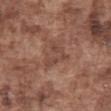biopsy status: total-body-photography surveillance lesion; no biopsy | site: the abdomen | image source: ~15 mm tile from a whole-body skin photo | TBP lesion metrics: an area of roughly 6 mm², an eccentricity of roughly 0.35, and a shape-asymmetry score of about 0.55 (0 = symmetric); an automated nevus-likeness rating near 0 out of 100 and a detector confidence of about 95 out of 100 that the crop contains a lesion | size: ~3.5 mm (longest diameter) | lighting: white-light | subject: male, approximately 75 years of age.